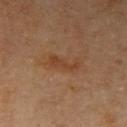<lesion>
<biopsy_status>not biopsied; imaged during a skin examination</biopsy_status>
<automated_metrics>
  <eccentricity>0.9</eccentricity>
  <vs_skin_darker_L>6.0</vs_skin_darker_L>
  <vs_skin_contrast_norm>6.0</vs_skin_contrast_norm>
  <border_irregularity_0_10>4.5</border_irregularity_0_10>
  <color_variation_0_10>2.0</color_variation_0_10>
  <peripheral_color_asymmetry>0.5</peripheral_color_asymmetry>
  <nevus_likeness_0_100>5</nevus_likeness_0_100>
  <lesion_detection_confidence_0_100>100</lesion_detection_confidence_0_100>
</automated_metrics>
<site>right upper arm</site>
<image>
  <source>total-body photography crop</source>
  <field_of_view_mm>15</field_of_view_mm>
</image>
<lesion_size>
  <long_diameter_mm_approx>4.0</long_diameter_mm_approx>
</lesion_size>
<patient>
  <sex>male</sex>
  <age_approx>65</age_approx>
</patient>
<lighting>cross-polarized</lighting>
</lesion>